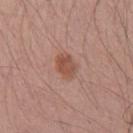Q: Was a biopsy performed?
A: catalogued during a skin exam; not biopsied
Q: Where on the body is the lesion?
A: the arm
Q: What are the patient's age and sex?
A: male, about 40 years old
Q: How was this image acquired?
A: ~15 mm crop, total-body skin-cancer survey
Q: Illumination type?
A: white-light
Q: Automated lesion metrics?
A: a lesion area of about 6 mm², an eccentricity of roughly 0.6, and two-axis asymmetry of about 0.25